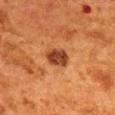This lesion was catalogued during total-body skin photography and was not selected for biopsy. The lesion is located on the mid back. A female subject, aged approximately 50. About 3.5 mm across. This image is a 15 mm lesion crop taken from a total-body photograph.